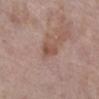workup: no biopsy performed (imaged during a skin exam); body site: the right lower leg; imaging modality: ~15 mm tile from a whole-body skin photo; patient: female, aged 63–67.